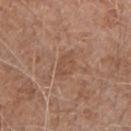<lesion>
  <biopsy_status>not biopsied; imaged during a skin examination</biopsy_status>
  <lesion_size>
    <long_diameter_mm_approx>3.0</long_diameter_mm_approx>
  </lesion_size>
  <patient>
    <sex>male</sex>
    <age_approx>60</age_approx>
  </patient>
  <automated_metrics>
    <vs_skin_contrast_norm>5.0</vs_skin_contrast_norm>
  </automated_metrics>
  <image>
    <source>total-body photography crop</source>
    <field_of_view_mm>15</field_of_view_mm>
  </image>
  <lighting>white-light</lighting>
  <site>left upper arm</site>
</lesion>